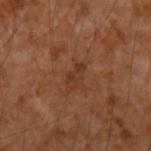Automated tile analysis of the lesion measured an average lesion color of about L≈36 a*≈23 b*≈31 (CIELAB), roughly 6 lightness units darker than nearby skin, and a normalized border contrast of about 5.5.
A male subject, aged approximately 55.
On the left forearm.
This is a cross-polarized tile.
About 3 mm across.
A lesion tile, about 15 mm wide, cut from a 3D total-body photograph.